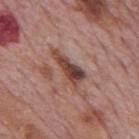| field | value |
|---|---|
| image | ~15 mm crop, total-body skin-cancer survey |
| patient | male, aged 73 to 77 |
| automated lesion analysis | border irregularity of about 6 on a 0–10 scale, internal color variation of about 7 on a 0–10 scale, and radial color variation of about 2 |
| illumination | white-light |
| lesion size | about 5.5 mm |
| body site | the mid back |